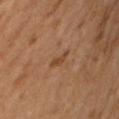{
  "biopsy_status": "not biopsied; imaged during a skin examination",
  "image": {
    "source": "total-body photography crop",
    "field_of_view_mm": 15
  },
  "lesion_size": {
    "long_diameter_mm_approx": 2.5
  },
  "lighting": "cross-polarized",
  "patient": {
    "sex": "male",
    "age_approx": 65
  }
}